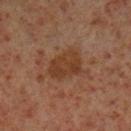follow-up: imaged on a skin check; not biopsied | anatomic site: the left lower leg | acquisition: ~15 mm tile from a whole-body skin photo | lesion diameter: about 4.5 mm | illumination: cross-polarized illumination | subject: male, aged approximately 60.